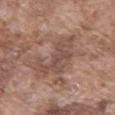This lesion was catalogued during total-body skin photography and was not selected for biopsy.
Imaged with white-light lighting.
A close-up tile cropped from a whole-body skin photograph, about 15 mm across.
From the mid back.
A male subject in their mid- to late 70s.